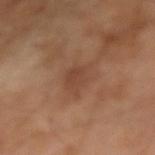Clinical impression:
This lesion was catalogued during total-body skin photography and was not selected for biopsy.
Image and clinical context:
The lesion is located on the right forearm. A male patient roughly 65 years of age. This image is a 15 mm lesion crop taken from a total-body photograph.